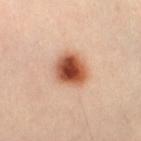Impression:
Part of a total-body skin-imaging series; this lesion was reviewed on a skin check and was not flagged for biopsy.
Context:
Longest diameter approximately 4 mm. A male patient about 40 years old. On the right thigh. An algorithmic analysis of the crop reported a lesion area of about 12 mm² and an eccentricity of roughly 0.45. A 15 mm close-up tile from a total-body photography series done for melanoma screening. Captured under cross-polarized illumination.